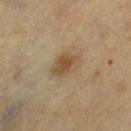Clinical impression:
Recorded during total-body skin imaging; not selected for excision or biopsy.
Background:
The patient is a female in their mid- to late 50s. Cropped from a total-body skin-imaging series; the visible field is about 15 mm. Captured under cross-polarized illumination. The lesion is on the leg.